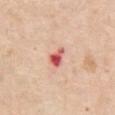Q: Was this lesion biopsied?
A: imaged on a skin check; not biopsied
Q: What did automated image analysis measure?
A: a lesion area of about 4 mm² and an outline eccentricity of about 0.75 (0 = round, 1 = elongated); an average lesion color of about L≈60 a*≈34 b*≈29 (CIELAB) and about 16 CIELAB-L* units darker than the surrounding skin; a border-irregularity rating of about 3/10, a color-variation rating of about 6/10, and peripheral color asymmetry of about 2
Q: How was the tile lit?
A: white-light
Q: What is the lesion's diameter?
A: about 3 mm
Q: What is the imaging modality?
A: ~15 mm crop, total-body skin-cancer survey
Q: What is the anatomic site?
A: the chest
Q: What are the patient's age and sex?
A: female, roughly 65 years of age